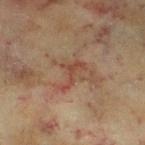This lesion was catalogued during total-body skin photography and was not selected for biopsy. The patient is a female about 60 years old. The lesion is located on the leg. About 4 mm across. A lesion tile, about 15 mm wide, cut from a 3D total-body photograph. Captured under cross-polarized illumination.